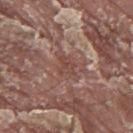Q: Was a biopsy performed?
A: no biopsy performed (imaged during a skin exam)
Q: Who is the patient?
A: male, roughly 40 years of age
Q: How was the tile lit?
A: white-light
Q: What kind of image is this?
A: 15 mm crop, total-body photography
Q: What is the lesion's diameter?
A: ≈2.5 mm
Q: Where on the body is the lesion?
A: the upper back